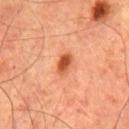An algorithmic analysis of the crop reported a symmetry-axis asymmetry near 0.2. The analysis additionally found an average lesion color of about L≈49 a*≈30 b*≈38 (CIELAB) and a lesion–skin lightness drop of about 14. And it measured border irregularity of about 2 on a 0–10 scale, internal color variation of about 4 on a 0–10 scale, and a peripheral color-asymmetry measure near 1. The software also gave lesion-presence confidence of about 100/100.
Imaged with cross-polarized lighting.
The lesion's longest dimension is about 3 mm.
The subject is a male roughly 65 years of age.
The lesion is on the chest.
Cropped from a whole-body photographic skin survey; the tile spans about 15 mm.